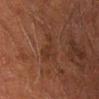follow-up — catalogued during a skin exam; not biopsied
image — total-body-photography crop, ~15 mm field of view
size — ≈3.5 mm
body site — the arm
patient — male, aged 58–62
TBP lesion metrics — a mean CIELAB color near L≈24 a*≈16 b*≈22, a lesion–skin lightness drop of about 4, and a normalized border contrast of about 4.5; a classifier nevus-likeness of about 0/100 and a detector confidence of about 100 out of 100 that the crop contains a lesion